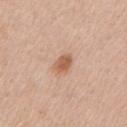Q: Is there a histopathology result?
A: no biopsy performed (imaged during a skin exam)
Q: How large is the lesion?
A: ~2.5 mm (longest diameter)
Q: How was the tile lit?
A: white-light
Q: Who is the patient?
A: female, in their mid- to late 40s
Q: How was this image acquired?
A: 15 mm crop, total-body photography
Q: Where on the body is the lesion?
A: the left upper arm